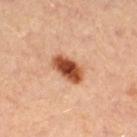The lesion was tiled from a total-body skin photograph and was not biopsied.
Imaged with cross-polarized lighting.
Approximately 4 mm at its widest.
The total-body-photography lesion software estimated an area of roughly 8.5 mm², a shape eccentricity near 0.85, and a symmetry-axis asymmetry near 0.15. The analysis additionally found an average lesion color of about L≈49 a*≈27 b*≈36 (CIELAB) and roughly 19 lightness units darker than nearby skin. The software also gave a border-irregularity index near 1.5/10, a within-lesion color-variation index near 5.5/10, and peripheral color asymmetry of about 1.5.
A female patient, approximately 45 years of age.
Cropped from a whole-body photographic skin survey; the tile spans about 15 mm.
From the leg.